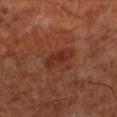notes: no biopsy performed (imaged during a skin exam); site: the leg; diameter: ≈3.5 mm; subject: male, approximately 70 years of age; imaging modality: total-body-photography crop, ~15 mm field of view; tile lighting: cross-polarized.